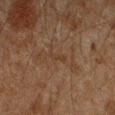| feature | finding |
|---|---|
| follow-up | total-body-photography surveillance lesion; no biopsy |
| body site | the left forearm |
| automated lesion analysis | a footprint of about 1.5 mm², a shape eccentricity near 0.95, and a shape-asymmetry score of about 0.6 (0 = symmetric); an average lesion color of about L≈30 a*≈13 b*≈25 (CIELAB), a lesion–skin lightness drop of about 4, and a normalized border contrast of about 5; a color-variation rating of about 0/10 and a peripheral color-asymmetry measure near 0; a nevus-likeness score of about 0/100 and a detector confidence of about 55 out of 100 that the crop contains a lesion |
| image | ~15 mm tile from a whole-body skin photo |
| lesion size | ~2.5 mm (longest diameter) |
| patient | male, aged 43 to 47 |